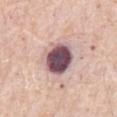Imaged during a routine full-body skin examination; the lesion was not biopsied and no histopathology is available. A lesion tile, about 15 mm wide, cut from a 3D total-body photograph. This is a white-light tile. A male patient, about 80 years old. Longest diameter approximately 4.5 mm. Located on the abdomen.